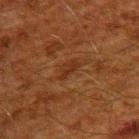Background: On the upper back. The subject is a male aged 58 to 62. Automated image analysis of the tile measured an area of roughly 2.5 mm², an outline eccentricity of about 0.9 (0 = round, 1 = elongated), and two-axis asymmetry of about 0.3. It also reported a lesion color around L≈24 a*≈20 b*≈27 in CIELAB and a lesion-to-skin contrast of about 6.5 (normalized; higher = more distinct). It also reported a border-irregularity index near 3.5/10, a within-lesion color-variation index near 0/10, and a peripheral color-asymmetry measure near 0. It also reported a nevus-likeness score of about 0/100 and lesion-presence confidence of about 95/100. A roughly 15 mm field-of-view crop from a total-body skin photograph. Approximately 2.5 mm at its widest.